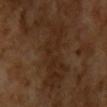workup = imaged on a skin check; not biopsied
image-analysis metrics = a nevus-likeness score of about 0/100 and a lesion-detection confidence of about 65/100
acquisition = total-body-photography crop, ~15 mm field of view
lesion diameter = ≈7.5 mm
lighting = cross-polarized illumination
patient = male, in their mid- to late 60s